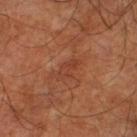workup = no biopsy performed (imaged during a skin exam)
location = the left lower leg
image = ~15 mm crop, total-body skin-cancer survey
patient = male, aged 58 to 62